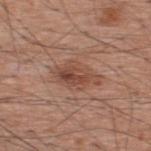Notes:
* biopsy status — total-body-photography surveillance lesion; no biopsy